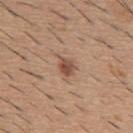<lesion>
  <biopsy_status>not biopsied; imaged during a skin examination</biopsy_status>
  <lesion_size>
    <long_diameter_mm_approx>2.5</long_diameter_mm_approx>
  </lesion_size>
  <patient>
    <sex>male</sex>
    <age_approx>60</age_approx>
  </patient>
  <site>mid back</site>
  <image>
    <source>total-body photography crop</source>
    <field_of_view_mm>15</field_of_view_mm>
  </image>
  <lighting>white-light</lighting>
</lesion>